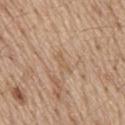Assessment: Imaged during a routine full-body skin examination; the lesion was not biopsied and no histopathology is available. Acquisition and patient details: From the mid back. A 15 mm crop from a total-body photograph taken for skin-cancer surveillance. An algorithmic analysis of the crop reported a footprint of about 2 mm², a shape eccentricity near 0.95, and a symmetry-axis asymmetry near 0.5. Measured at roughly 2.5 mm in maximum diameter. This is a white-light tile. A male subject, aged 68 to 72.